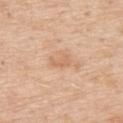Captured during whole-body skin photography for melanoma surveillance; the lesion was not biopsied. A male subject about 60 years old. The lesion's longest dimension is about 2.5 mm. From the upper back. A 15 mm close-up extracted from a 3D total-body photography capture. Automated image analysis of the tile measured an area of roughly 3 mm², a shape eccentricity near 0.8, and two-axis asymmetry of about 0.5. The software also gave roughly 7 lightness units darker than nearby skin and a normalized lesion–skin contrast near 5. Imaged with white-light lighting.